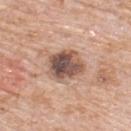Q: Is there a histopathology result?
A: imaged on a skin check; not biopsied
Q: What is the lesion's diameter?
A: ≈4 mm
Q: What kind of image is this?
A: total-body-photography crop, ~15 mm field of view
Q: What did automated image analysis measure?
A: an average lesion color of about L≈51 a*≈18 b*≈25 (CIELAB) and roughly 17 lightness units darker than nearby skin; an automated nevus-likeness rating near 0 out of 100 and a detector confidence of about 100 out of 100 that the crop contains a lesion
Q: Lesion location?
A: the upper back
Q: What lighting was used for the tile?
A: white-light
Q: Who is the patient?
A: male, aged 78 to 82